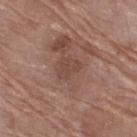workup=total-body-photography surveillance lesion; no biopsy
size=about 3 mm
patient=female, roughly 70 years of age
image=15 mm crop, total-body photography
lighting=white-light illumination
anatomic site=the leg